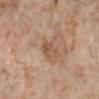Clinical impression:
Part of a total-body skin-imaging series; this lesion was reviewed on a skin check and was not flagged for biopsy.